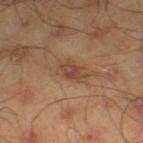Imaged during a routine full-body skin examination; the lesion was not biopsied and no histopathology is available. A male patient aged around 45. This is a cross-polarized tile. The lesion's longest dimension is about 3.5 mm. On the left thigh. A region of skin cropped from a whole-body photographic capture, roughly 15 mm wide.